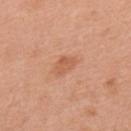Notes:
* follow-up: catalogued during a skin exam; not biopsied
* tile lighting: white-light illumination
* anatomic site: the upper back
* acquisition: total-body-photography crop, ~15 mm field of view
* patient: male, in their 70s
* size: about 3 mm